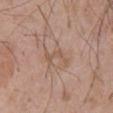Recorded during total-body skin imaging; not selected for excision or biopsy.
A 15 mm close-up tile from a total-body photography series done for melanoma screening.
The subject is a male about 60 years old.
The lesion is located on the chest.
Longest diameter approximately 3.5 mm.
This is a white-light tile.